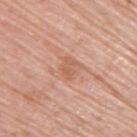Assessment:
This lesion was catalogued during total-body skin photography and was not selected for biopsy.
Image and clinical context:
A male subject aged around 80. The lesion is on the right upper arm. A roughly 15 mm field-of-view crop from a total-body skin photograph.